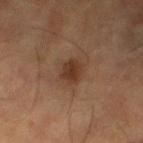The lesion was tiled from a total-body skin photograph and was not biopsied.
Automated tile analysis of the lesion measured a lesion area of about 5 mm². The software also gave an average lesion color of about L≈30 a*≈17 b*≈26 (CIELAB) and a normalized lesion–skin contrast near 8.5. The software also gave lesion-presence confidence of about 100/100.
Located on the left lower leg.
This is a cross-polarized tile.
A close-up tile cropped from a whole-body skin photograph, about 15 mm across.
A female subject, aged 53–57.